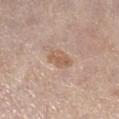notes: catalogued during a skin exam; not biopsied | patient: female, aged 63–67 | acquisition: total-body-photography crop, ~15 mm field of view | size: about 3.5 mm | lighting: white-light | site: the right lower leg.